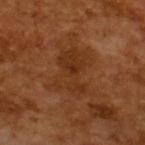The lesion was tiled from a total-body skin photograph and was not biopsied. A 15 mm crop from a total-body photograph taken for skin-cancer surveillance. A male subject, aged 63 to 67.